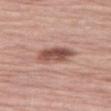biopsy_status: not biopsied; imaged during a skin examination
image:
  source: total-body photography crop
  field_of_view_mm: 15
patient:
  sex: female
  age_approx: 70
automated_metrics:
  nevus_likeness_0_100: 85
  lesion_detection_confidence_0_100: 100
site: right thigh
lighting: white-light